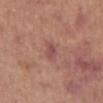Recorded during total-body skin imaging; not selected for excision or biopsy.
The total-body-photography lesion software estimated roughly 8 lightness units darker than nearby skin.
A female patient about 65 years old.
The lesion is located on the arm.
Cropped from a whole-body photographic skin survey; the tile spans about 15 mm.
Captured under white-light illumination.